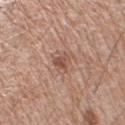{
  "biopsy_status": "not biopsied; imaged during a skin examination",
  "site": "right upper arm",
  "lesion_size": {
    "long_diameter_mm_approx": 3.0
  },
  "automated_metrics": {
    "cielab_L": 52,
    "cielab_a": 21,
    "cielab_b": 27,
    "vs_skin_contrast_norm": 6.5,
    "nevus_likeness_0_100": 5,
    "lesion_detection_confidence_0_100": 100
  },
  "image": {
    "source": "total-body photography crop",
    "field_of_view_mm": 15
  },
  "patient": {
    "sex": "male",
    "age_approx": 65
  },
  "lighting": "white-light"
}